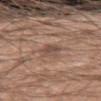subject: male, in their 20s; anatomic site: the left upper arm; diameter: ~3 mm (longest diameter); image source: 15 mm crop, total-body photography; lighting: white-light illumination.